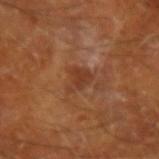Case summary:
• notes: imaged on a skin check; not biopsied
• location: the left forearm
• subject: male, aged around 65
• acquisition: ~15 mm tile from a whole-body skin photo